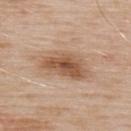From the upper back. A male subject, aged 53 to 57. About 6 mm across. This image is a 15 mm lesion crop taken from a total-body photograph. This is a white-light tile.